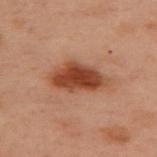Imaged during a routine full-body skin examination; the lesion was not biopsied and no histopathology is available. A female subject, aged 53 to 57. The lesion is located on the upper back. Longest diameter approximately 6 mm. Captured under cross-polarized illumination. A region of skin cropped from a whole-body photographic capture, roughly 15 mm wide. An algorithmic analysis of the crop reported an outline eccentricity of about 0.85 (0 = round, 1 = elongated) and two-axis asymmetry of about 0.2. It also reported border irregularity of about 2.5 on a 0–10 scale, a within-lesion color-variation index near 3.5/10, and peripheral color asymmetry of about 1.